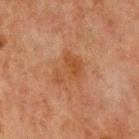Case summary:
* follow-up · catalogued during a skin exam; not biopsied
* patient · male, roughly 65 years of age
* body site · the chest
* image source · 15 mm crop, total-body photography
* automated metrics · an eccentricity of roughly 0.65 and a shape-asymmetry score of about 0.45 (0 = symmetric); an average lesion color of about L≈40 a*≈21 b*≈31 (CIELAB), about 6 CIELAB-L* units darker than the surrounding skin, and a lesion-to-skin contrast of about 6 (normalized; higher = more distinct)
* lesion diameter · about 4 mm
* lighting · cross-polarized